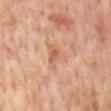notes: imaged on a skin check; not biopsied
patient: male, about 65 years old
body site: the mid back
acquisition: 15 mm crop, total-body photography